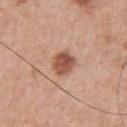Captured during whole-body skin photography for melanoma surveillance; the lesion was not biopsied.